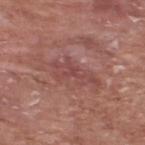A lesion tile, about 15 mm wide, cut from a 3D total-body photograph. A male patient approximately 75 years of age. Located on the upper back. Approximately 5.5 mm at its widest. Imaged with white-light lighting.